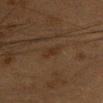biopsy_status: not biopsied; imaged during a skin examination
patient:
  sex: female
  age_approx: 40
site: chest
image:
  source: total-body photography crop
  field_of_view_mm: 15